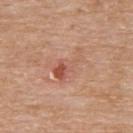The lesion was tiled from a total-body skin photograph and was not biopsied. About 4.5 mm across. An algorithmic analysis of the crop reported a normalized lesion–skin contrast near 5.5. This is a white-light tile. A region of skin cropped from a whole-body photographic capture, roughly 15 mm wide. A female patient, roughly 75 years of age. From the upper back.